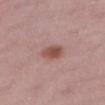lesion_size:
  long_diameter_mm_approx: 3.0
site: right thigh
lighting: white-light
automated_metrics:
  area_mm2_approx: 5.5
  border_irregularity_0_10: 1.5
  color_variation_0_10: 3.5
patient:
  sex: female
  age_approx: 45
image:
  source: total-body photography crop
  field_of_view_mm: 15